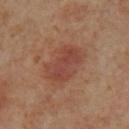biopsy_status: not biopsied; imaged during a skin examination
lighting: cross-polarized
lesion_size:
  long_diameter_mm_approx: 5.0
patient:
  sex: female
  age_approx: 55
image:
  source: total-body photography crop
  field_of_view_mm: 15
automated_metrics:
  area_mm2_approx: 12.0
  eccentricity: 0.8
  shape_asymmetry: 0.25
  cielab_L: 45
  cielab_a: 25
  cielab_b: 28
  vs_skin_contrast_norm: 6.5
  border_irregularity_0_10: 3.0
  color_variation_0_10: 3.5
  peripheral_color_asymmetry: 1.0
site: leg